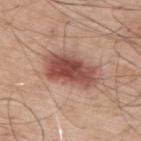• biopsy status — total-body-photography surveillance lesion; no biopsy
• body site — the upper back
• illumination — white-light
• acquisition — ~15 mm tile from a whole-body skin photo
• size — about 7 mm
• patient — male, approximately 70 years of age